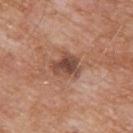| key | value |
|---|---|
| follow-up | catalogued during a skin exam; not biopsied |
| lesion diameter | ≈3.5 mm |
| patient | male, aged 78 to 82 |
| imaging modality | 15 mm crop, total-body photography |
| lighting | white-light illumination |
| location | the upper back |
| automated metrics | a footprint of about 8 mm² and a shape eccentricity near 0.45; a mean CIELAB color near L≈47 a*≈21 b*≈28 and a lesion–skin lightness drop of about 12; a color-variation rating of about 5.5/10 |